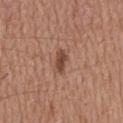  biopsy_status: not biopsied; imaged during a skin examination
  site: mid back
  automated_metrics:
    border_irregularity_0_10: 2.5
    color_variation_0_10: 1.5
    peripheral_color_asymmetry: 0.5
    nevus_likeness_0_100: 90
    lesion_detection_confidence_0_100: 100
  patient:
    sex: male
    age_approx: 65
  lighting: white-light
  image:
    source: total-body photography crop
    field_of_view_mm: 15
  lesion_size:
    long_diameter_mm_approx: 3.0